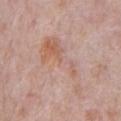Recorded during total-body skin imaging; not selected for excision or biopsy. Approximately 9 mm at its widest. The patient is a male about 70 years old. The total-body-photography lesion software estimated a mean CIELAB color near L≈61 a*≈20 b*≈27 and a normalized lesion–skin contrast near 4.5. It also reported a border-irregularity index near 8.5/10, internal color variation of about 8 on a 0–10 scale, and radial color variation of about 3. On the chest. Captured under white-light illumination. A roughly 15 mm field-of-view crop from a total-body skin photograph.